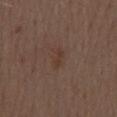The lesion was tiled from a total-body skin photograph and was not biopsied. From the mid back. Captured under white-light illumination. The lesion's longest dimension is about 2.5 mm. Automated tile analysis of the lesion measured a color-variation rating of about 0/10 and radial color variation of about 0. A region of skin cropped from a whole-body photographic capture, roughly 15 mm wide. The patient is a male aged 68 to 72.